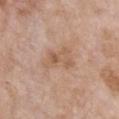Imaged during a routine full-body skin examination; the lesion was not biopsied and no histopathology is available. Captured under white-light illumination. The lesion is on the chest. A female subject approximately 75 years of age. A 15 mm close-up extracted from a 3D total-body photography capture.